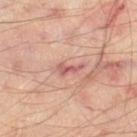{"biopsy_status": "not biopsied; imaged during a skin examination", "lighting": "cross-polarized", "site": "leg", "lesion_size": {"long_diameter_mm_approx": 3.0}, "image": {"source": "total-body photography crop", "field_of_view_mm": 15}, "patient": {"age_approx": 55}}